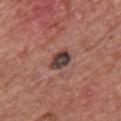Q: Is there a histopathology result?
A: imaged on a skin check; not biopsied
Q: Lesion location?
A: the chest
Q: What is the imaging modality?
A: ~15 mm tile from a whole-body skin photo
Q: What did automated image analysis measure?
A: roughly 15 lightness units darker than nearby skin and a lesion-to-skin contrast of about 12 (normalized; higher = more distinct)
Q: How was the tile lit?
A: white-light
Q: How large is the lesion?
A: ≈3 mm
Q: What are the patient's age and sex?
A: male, in their mid- to late 70s